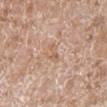Q: Was a biopsy performed?
A: no biopsy performed (imaged during a skin exam)
Q: Who is the patient?
A: male, about 60 years old
Q: What is the imaging modality?
A: 15 mm crop, total-body photography
Q: Where on the body is the lesion?
A: the left lower leg
Q: How large is the lesion?
A: about 3 mm
Q: Automated lesion metrics?
A: an eccentricity of roughly 0.95 and two-axis asymmetry of about 0.4; a mean CIELAB color near L≈60 a*≈19 b*≈30; a nevus-likeness score of about 0/100 and a lesion-detection confidence of about 85/100